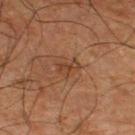Findings:
- workup · imaged on a skin check; not biopsied
- tile lighting · cross-polarized illumination
- automated lesion analysis · border irregularity of about 4.5 on a 0–10 scale, a within-lesion color-variation index near 1/10, and a peripheral color-asymmetry measure near 0.5
- acquisition · 15 mm crop, total-body photography
- subject · male, about 65 years old
- anatomic site · the left lower leg
- lesion size · about 2.5 mm